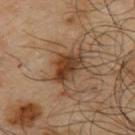Captured during whole-body skin photography for melanoma surveillance; the lesion was not biopsied.
A male subject about 65 years old.
Automated tile analysis of the lesion measured a lesion color around L≈37 a*≈18 b*≈29 in CIELAB, a lesion–skin lightness drop of about 11, and a lesion-to-skin contrast of about 9.5 (normalized; higher = more distinct). It also reported a classifier nevus-likeness of about 75/100 and a lesion-detection confidence of about 100/100.
Longest diameter approximately 4.5 mm.
A close-up tile cropped from a whole-body skin photograph, about 15 mm across.
Captured under cross-polarized illumination.
The lesion is on the upper back.